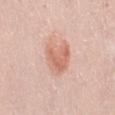Notes:
– workup — imaged on a skin check; not biopsied
– patient — female, in their mid- to late 60s
– imaging modality — ~15 mm crop, total-body skin-cancer survey
– location — the abdomen